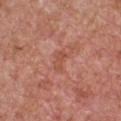Context: The recorded lesion diameter is about 2.5 mm. A male subject, in their mid- to late 60s. The lesion is on the chest. The tile uses white-light illumination. A 15 mm crop from a total-body photograph taken for skin-cancer surveillance.